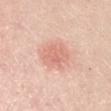workup — no biopsy performed (imaged during a skin exam); illumination — white-light; acquisition — total-body-photography crop, ~15 mm field of view; body site — the right upper arm; subject — male, roughly 50 years of age.